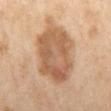This lesion was catalogued during total-body skin photography and was not selected for biopsy. The lesion is located on the mid back. An algorithmic analysis of the crop reported a mean CIELAB color near L≈50 a*≈17 b*≈30, roughly 10 lightness units darker than nearby skin, and a normalized lesion–skin contrast near 7.5. The patient is a female aged approximately 60. A lesion tile, about 15 mm wide, cut from a 3D total-body photograph. Measured at roughly 7 mm in maximum diameter. Captured under cross-polarized illumination.